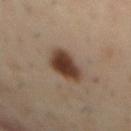Assessment: The lesion was photographed on a routine skin check and not biopsied; there is no pathology result. Background: A male patient aged approximately 55. From the mid back. Cropped from a whole-body photographic skin survey; the tile spans about 15 mm.